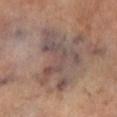Impression: Part of a total-body skin-imaging series; this lesion was reviewed on a skin check and was not flagged for biopsy. Clinical summary: An algorithmic analysis of the crop reported an automated nevus-likeness rating near 0 out of 100 and lesion-presence confidence of about 55/100. A 15 mm close-up tile from a total-body photography series done for melanoma screening. Longest diameter approximately 8 mm. The tile uses cross-polarized illumination. A male subject, aged 68 to 72. Located on the left lower leg.